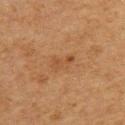Q: Is there a histopathology result?
A: no biopsy performed (imaged during a skin exam)